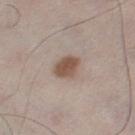workup: no biopsy performed (imaged during a skin exam) | image source: 15 mm crop, total-body photography | automated lesion analysis: a lesion color around L≈52 a*≈16 b*≈26 in CIELAB, about 12 CIELAB-L* units darker than the surrounding skin, and a lesion-to-skin contrast of about 9 (normalized; higher = more distinct); a border-irregularity rating of about 1.5/10, internal color variation of about 2.5 on a 0–10 scale, and peripheral color asymmetry of about 1; an automated nevus-likeness rating near 100 out of 100 and lesion-presence confidence of about 100/100 | patient: male, aged 43–47 | lesion diameter: ≈3.5 mm | lighting: white-light illumination | site: the left thigh.